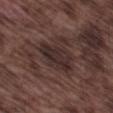Clinical impression:
The lesion was tiled from a total-body skin photograph and was not biopsied.
Acquisition and patient details:
The lesion-visualizer software estimated an area of roughly 12 mm², an eccentricity of roughly 0.8, and a shape-asymmetry score of about 0.25 (0 = symmetric). Located on the left thigh. Approximately 5.5 mm at its widest. A male patient, about 75 years old. Captured under white-light illumination. Cropped from a whole-body photographic skin survey; the tile spans about 15 mm.